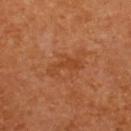| key | value |
|---|---|
| follow-up | imaged on a skin check; not biopsied |
| illumination | cross-polarized illumination |
| subject | female, aged 63–67 |
| location | the back |
| imaging modality | ~15 mm crop, total-body skin-cancer survey |